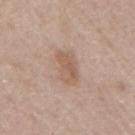Q: Is there a histopathology result?
A: catalogued during a skin exam; not biopsied
Q: What is the imaging modality?
A: ~15 mm tile from a whole-body skin photo
Q: Where on the body is the lesion?
A: the right upper arm
Q: What are the patient's age and sex?
A: female, roughly 60 years of age